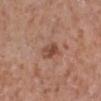  biopsy_status: not biopsied; imaged during a skin examination
  site: right lower leg
  image:
    source: total-body photography crop
    field_of_view_mm: 15
  lighting: white-light
  automated_metrics:
    area_mm2_approx: 4.5
    shape_asymmetry: 0.2
    vs_skin_contrast_norm: 7.5
    nevus_likeness_0_100: 35
    lesion_detection_confidence_0_100: 100
  patient:
    sex: male
    age_approx: 75
  lesion_size:
    long_diameter_mm_approx: 3.0